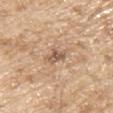<case>
<biopsy_status>not biopsied; imaged during a skin examination</biopsy_status>
<automated_metrics>
  <eccentricity>0.75</eccentricity>
  <shape_asymmetry>0.3</shape_asymmetry>
  <cielab_L>56</cielab_L>
  <cielab_a>18</cielab_a>
  <cielab_b>29</cielab_b>
  <vs_skin_contrast_norm>8.0</vs_skin_contrast_norm>
  <color_variation_0_10>3.0</color_variation_0_10>
  <peripheral_color_asymmetry>1.0</peripheral_color_asymmetry>
  <nevus_likeness_0_100>0</nevus_likeness_0_100>
</automated_metrics>
<lighting>white-light</lighting>
<patient>
  <sex>male</sex>
  <age_approx>70</age_approx>
</patient>
<image>
  <source>total-body photography crop</source>
  <field_of_view_mm>15</field_of_view_mm>
</image>
<site>left upper arm</site>
<lesion_size>
  <long_diameter_mm_approx>2.5</long_diameter_mm_approx>
</lesion_size>
</case>